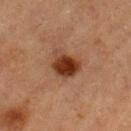Captured during whole-body skin photography for melanoma surveillance; the lesion was not biopsied. A male patient, aged around 85 or older. Imaged with cross-polarized lighting. About 3.5 mm across. An algorithmic analysis of the crop reported border irregularity of about 2.5 on a 0–10 scale and peripheral color asymmetry of about 2. A 15 mm close-up extracted from a 3D total-body photography capture. The lesion is on the left thigh.